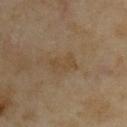Captured during whole-body skin photography for melanoma surveillance; the lesion was not biopsied. The total-body-photography lesion software estimated a footprint of about 5 mm², an eccentricity of roughly 0.9, and a symmetry-axis asymmetry near 0.35. The analysis additionally found a mean CIELAB color near L≈43 a*≈12 b*≈31 and a lesion-to-skin contrast of about 5.5 (normalized; higher = more distinct). The analysis additionally found a lesion-detection confidence of about 100/100. A 15 mm close-up tile from a total-body photography series done for melanoma screening. Located on the upper back. The subject is a female aged 33–37.